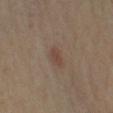Findings:
- biopsy status: total-body-photography surveillance lesion; no biopsy
- TBP lesion metrics: a lesion color around L≈40 a*≈16 b*≈23 in CIELAB, roughly 7 lightness units darker than nearby skin, and a normalized border contrast of about 6; an automated nevus-likeness rating near 45 out of 100 and a detector confidence of about 100 out of 100 that the crop contains a lesion
- body site: the left upper arm
- image source: total-body-photography crop, ~15 mm field of view
- patient: male, about 70 years old
- diameter: ~2.5 mm (longest diameter)